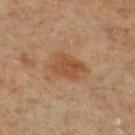No biopsy was performed on this lesion — it was imaged during a full skin examination and was not determined to be concerning. Approximately 4.5 mm at its widest. A 15 mm crop from a total-body photograph taken for skin-cancer surveillance. The lesion-visualizer software estimated a footprint of about 11 mm², an eccentricity of roughly 0.75, and two-axis asymmetry of about 0.2. A male subject, roughly 60 years of age. Located on the right lower leg. This is a cross-polarized tile.